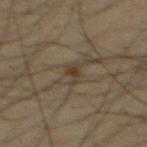Clinical impression:
This lesion was catalogued during total-body skin photography and was not selected for biopsy.
Context:
Automated tile analysis of the lesion measured a footprint of about 3 mm², an eccentricity of roughly 0.8, and two-axis asymmetry of about 0.4. It also reported about 7 CIELAB-L* units darker than the surrounding skin and a normalized border contrast of about 7. The analysis additionally found a border-irregularity index near 4.5/10, internal color variation of about 1 on a 0–10 scale, and peripheral color asymmetry of about 0.5. The analysis additionally found a classifier nevus-likeness of about 10/100 and a lesion-detection confidence of about 95/100. Captured under cross-polarized illumination. From the mid back. A roughly 15 mm field-of-view crop from a total-body skin photograph. The patient is a male roughly 35 years of age. About 3 mm across.